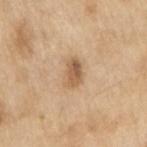The lesion is located on the right upper arm.
Longest diameter approximately 3 mm.
Imaged with white-light lighting.
A close-up tile cropped from a whole-body skin photograph, about 15 mm across.
A male patient, approximately 70 years of age.
The lesion-visualizer software estimated a shape-asymmetry score of about 0.15 (0 = symmetric). It also reported an average lesion color of about L≈59 a*≈17 b*≈35 (CIELAB), roughly 11 lightness units darker than nearby skin, and a normalized lesion–skin contrast near 7.5. The software also gave internal color variation of about 5.5 on a 0–10 scale and a peripheral color-asymmetry measure near 2. And it measured a classifier nevus-likeness of about 75/100 and lesion-presence confidence of about 100/100.